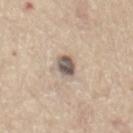The lesion was tiled from a total-body skin photograph and was not biopsied.